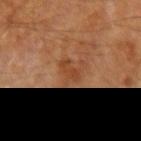This lesion was catalogued during total-body skin photography and was not selected for biopsy.
Captured under cross-polarized illumination.
The total-body-photography lesion software estimated an area of roughly 4 mm², an eccentricity of roughly 0.85, and two-axis asymmetry of about 0.4. And it measured a border-irregularity index near 4/10, a within-lesion color-variation index near 2/10, and a peripheral color-asymmetry measure near 0.5.
A male subject, about 85 years old or older.
A region of skin cropped from a whole-body photographic capture, roughly 15 mm wide.
The lesion's longest dimension is about 3 mm.
The lesion is located on the right forearm.